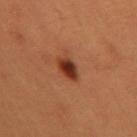notes: total-body-photography surveillance lesion; no biopsy
illumination: cross-polarized illumination
location: the right upper arm
diameter: ~3 mm (longest diameter)
imaging modality: total-body-photography crop, ~15 mm field of view
subject: female, approximately 40 years of age
automated lesion analysis: a footprint of about 5 mm² and an outline eccentricity of about 0.7 (0 = round, 1 = elongated); about 14 CIELAB-L* units darker than the surrounding skin and a normalized lesion–skin contrast near 11; a border-irregularity index near 2.5/10, a color-variation rating of about 5.5/10, and a peripheral color-asymmetry measure near 1.5